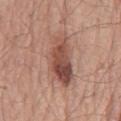Clinical impression: This lesion was catalogued during total-body skin photography and was not selected for biopsy. Clinical summary: The tile uses white-light illumination. A close-up tile cropped from a whole-body skin photograph, about 15 mm across. A male patient aged 68–72. Located on the mid back. The total-body-photography lesion software estimated an area of roughly 18 mm² and two-axis asymmetry of about 0.25. And it measured a mean CIELAB color near L≈50 a*≈22 b*≈26, roughly 12 lightness units darker than nearby skin, and a normalized lesion–skin contrast near 8.5.